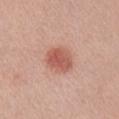Clinical impression:
Imaged during a routine full-body skin examination; the lesion was not biopsied and no histopathology is available.
Image and clinical context:
Captured under white-light illumination. A region of skin cropped from a whole-body photographic capture, roughly 15 mm wide. A male patient aged 53 to 57. Measured at roughly 4 mm in maximum diameter. The lesion-visualizer software estimated a mean CIELAB color near L≈57 a*≈26 b*≈29, about 11 CIELAB-L* units darker than the surrounding skin, and a normalized lesion–skin contrast near 7.5. Located on the right upper arm.